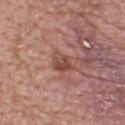The lesion was photographed on a routine skin check and not biopsied; there is no pathology result.
From the upper back.
The subject is a male roughly 55 years of age.
The total-body-photography lesion software estimated a footprint of about 5 mm², an eccentricity of roughly 0.6, and a shape-asymmetry score of about 0.25 (0 = symmetric).
About 3 mm across.
Imaged with white-light lighting.
A 15 mm close-up tile from a total-body photography series done for melanoma screening.